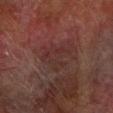follow-up = total-body-photography surveillance lesion; no biopsy | size = ~4.5 mm (longest diameter) | image source = total-body-photography crop, ~15 mm field of view | anatomic site = the right forearm | subject = male, about 75 years old | illumination = cross-polarized illumination.